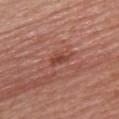No biopsy was performed on this lesion — it was imaged during a full skin examination and was not determined to be concerning.
Cropped from a whole-body photographic skin survey; the tile spans about 15 mm.
On the back.
A female patient aged 53 to 57.